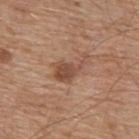Recorded during total-body skin imaging; not selected for excision or biopsy. This is a white-light tile. A 15 mm crop from a total-body photograph taken for skin-cancer surveillance. Located on the mid back. A male subject roughly 65 years of age.